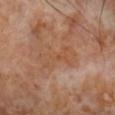workup: imaged on a skin check; not biopsied
lesion size: about 5 mm
subject: male, in their 70s
anatomic site: the right forearm
image: total-body-photography crop, ~15 mm field of view